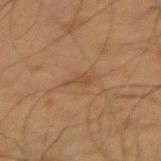No biopsy was performed on this lesion — it was imaged during a full skin examination and was not determined to be concerning. Longest diameter approximately 2.5 mm. A 15 mm crop from a total-body photograph taken for skin-cancer surveillance. The tile uses cross-polarized illumination. A male subject, aged 73 to 77. Automated tile analysis of the lesion measured a shape eccentricity near 0.9. The analysis additionally found an automated nevus-likeness rating near 0 out of 100. The lesion is located on the right thigh.